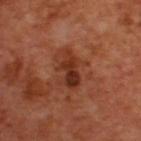<case>
<biopsy_status>not biopsied; imaged during a skin examination</biopsy_status>
</case>